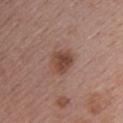  biopsy_status: not biopsied; imaged during a skin examination
  patient:
    sex: female
    age_approx: 55
  site: upper back
  image:
    source: total-body photography crop
    field_of_view_mm: 15
  lighting: white-light
  lesion_size:
    long_diameter_mm_approx: 3.0